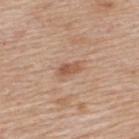| feature | finding |
|---|---|
| notes | no biopsy performed (imaged during a skin exam) |
| size | ≈2.5 mm |
| location | the upper back |
| image | 15 mm crop, total-body photography |
| illumination | white-light |
| patient | male, roughly 60 years of age |